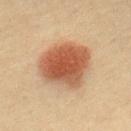workup = total-body-photography surveillance lesion; no biopsy | location = the back | lighting = cross-polarized | image source = 15 mm crop, total-body photography | subject = male, aged 38 to 42.